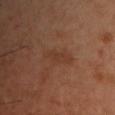Imaged during a routine full-body skin examination; the lesion was not biopsied and no histopathology is available.
A male patient aged approximately 40.
Measured at roughly 4.5 mm in maximum diameter.
Captured under cross-polarized illumination.
A 15 mm close-up tile from a total-body photography series done for melanoma screening.
The lesion-visualizer software estimated internal color variation of about 1 on a 0–10 scale and a peripheral color-asymmetry measure near 0.5. It also reported a nevus-likeness score of about 0/100 and a detector confidence of about 100 out of 100 that the crop contains a lesion.
Located on the chest.